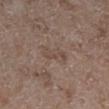Context: Longest diameter approximately 4 mm. The lesion is on the leg. Cropped from a whole-body photographic skin survey; the tile spans about 15 mm. Captured under white-light illumination. A female subject aged approximately 70.